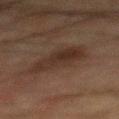{"biopsy_status": "not biopsied; imaged during a skin examination", "image": {"source": "total-body photography crop", "field_of_view_mm": 15}, "lesion_size": {"long_diameter_mm_approx": 5.5}, "automated_metrics": {"nevus_likeness_0_100": 25}, "lighting": "cross-polarized", "site": "back", "patient": {"sex": "male", "age_approx": 65}}